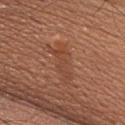The lesion was photographed on a routine skin check and not biopsied; there is no pathology result.
From the chest.
A male subject, aged approximately 60.
The lesion's longest dimension is about 5 mm.
A lesion tile, about 15 mm wide, cut from a 3D total-body photograph.
Automated tile analysis of the lesion measured a mean CIELAB color near L≈45 a*≈24 b*≈31, roughly 6 lightness units darker than nearby skin, and a normalized lesion–skin contrast near 5. It also reported border irregularity of about 8 on a 0–10 scale and radial color variation of about 0.5.
Captured under white-light illumination.